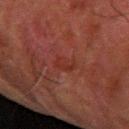biopsy status = imaged on a skin check; not biopsied
imaging modality = ~15 mm crop, total-body skin-cancer survey
body site = the left forearm
patient = male, roughly 80 years of age